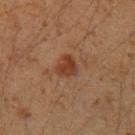Case summary:
* subject · male, in their 50s
* imaging modality · ~15 mm tile from a whole-body skin photo
* tile lighting · cross-polarized illumination
* size · ≈3 mm
* automated lesion analysis · a mean CIELAB color near L≈35 a*≈20 b*≈29, roughly 8 lightness units darker than nearby skin, and a normalized lesion–skin contrast near 8; a nevus-likeness score of about 80/100 and a lesion-detection confidence of about 100/100
* site · the left upper arm